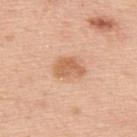Part of a total-body skin-imaging series; this lesion was reviewed on a skin check and was not flagged for biopsy.